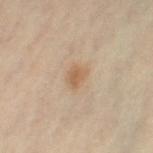No biopsy was performed on this lesion — it was imaged during a full skin examination and was not determined to be concerning. The lesion is located on the left thigh. A 15 mm close-up extracted from a 3D total-body photography capture. A female patient, in their mid-40s.